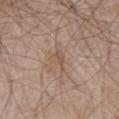notes=catalogued during a skin exam; not biopsied | size=about 3 mm | subject=male, in their mid-60s | image source=15 mm crop, total-body photography | body site=the abdomen | tile lighting=white-light illumination.